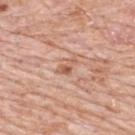A male patient aged 78–82. This image is a 15 mm lesion crop taken from a total-body photograph. Measured at roughly 3 mm in maximum diameter. An algorithmic analysis of the crop reported a footprint of about 3 mm² and two-axis asymmetry of about 0.55. The software also gave a normalized border contrast of about 7. Captured under white-light illumination. The lesion is on the upper back.